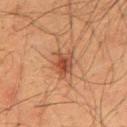automated lesion analysis = a border-irregularity rating of about 4/10 and peripheral color asymmetry of about 1.5; an automated nevus-likeness rating near 85 out of 100 and lesion-presence confidence of about 100/100 | body site = the mid back | tile lighting = cross-polarized | image source = ~15 mm crop, total-body skin-cancer survey | lesion size = ~3.5 mm (longest diameter) | patient = male, in their mid-30s.